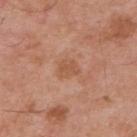Q: How was this image acquired?
A: ~15 mm tile from a whole-body skin photo
Q: Patient demographics?
A: male, in their mid- to late 50s
Q: Where on the body is the lesion?
A: the upper back